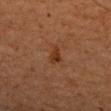| key | value |
|---|---|
| biopsy status | catalogued during a skin exam; not biopsied |